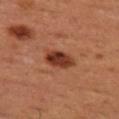Captured under cross-polarized illumination. Automated image analysis of the tile measured an area of roughly 7.5 mm² and a shape eccentricity near 0.8. The analysis additionally found border irregularity of about 1.5 on a 0–10 scale, a color-variation rating of about 5/10, and peripheral color asymmetry of about 2. It also reported an automated nevus-likeness rating near 95 out of 100 and a lesion-detection confidence of about 100/100. A male patient in their 50s. On the left thigh. Cropped from a whole-body photographic skin survey; the tile spans about 15 mm.